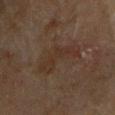  biopsy_status: not biopsied; imaged during a skin examination
  patient:
    sex: male
    age_approx: 70
  image:
    source: total-body photography crop
    field_of_view_mm: 15
  site: left upper arm
  lighting: cross-polarized